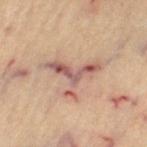{
  "biopsy_status": "not biopsied; imaged during a skin examination",
  "automated_metrics": {
    "area_mm2_approx": 10.0,
    "eccentricity": 0.7,
    "shape_asymmetry": 0.8,
    "vs_skin_darker_L": 12.0,
    "vs_skin_contrast_norm": 8.5
  },
  "patient": {
    "sex": "female",
    "age_approx": 65
  },
  "site": "left thigh",
  "image": {
    "source": "total-body photography crop",
    "field_of_view_mm": 15
  },
  "lesion_size": {
    "long_diameter_mm_approx": 6.0
  },
  "lighting": "cross-polarized"
}